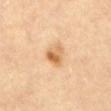Q: Is there a histopathology result?
A: total-body-photography surveillance lesion; no biopsy
Q: Lesion size?
A: ~3 mm (longest diameter)
Q: Illumination type?
A: cross-polarized
Q: Where on the body is the lesion?
A: the abdomen
Q: What kind of image is this?
A: ~15 mm crop, total-body skin-cancer survey
Q: What are the patient's age and sex?
A: female, aged around 65